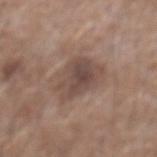<record>
<biopsy_status>not biopsied; imaged during a skin examination</biopsy_status>
<patient>
  <sex>male</sex>
  <age_approx>60</age_approx>
</patient>
<image>
  <source>total-body photography crop</source>
  <field_of_view_mm>15</field_of_view_mm>
</image>
<lighting>white-light</lighting>
<site>mid back</site>
</record>